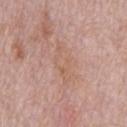Assessment: Recorded during total-body skin imaging; not selected for excision or biopsy. Clinical summary: A male subject, roughly 70 years of age. A roughly 15 mm field-of-view crop from a total-body skin photograph. On the chest. The tile uses white-light illumination. Automated tile analysis of the lesion measured an average lesion color of about L≈60 a*≈19 b*≈29 (CIELAB), roughly 5 lightness units darker than nearby skin, and a normalized border contrast of about 4.5. The analysis additionally found a border-irregularity rating of about 6/10, a color-variation rating of about 2.5/10, and radial color variation of about 0.5.